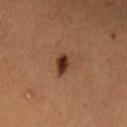notes — catalogued during a skin exam; not biopsied
anatomic site — the mid back
subject — male, aged around 50
tile lighting — cross-polarized
size — ~3 mm (longest diameter)
imaging modality — ~15 mm crop, total-body skin-cancer survey
automated lesion analysis — an area of roughly 5 mm², an eccentricity of roughly 0.7, and a shape-asymmetry score of about 0.3 (0 = symmetric); an average lesion color of about L≈28 a*≈18 b*≈24 (CIELAB) and about 10 CIELAB-L* units darker than the surrounding skin; a border-irregularity rating of about 3/10, a within-lesion color-variation index near 4.5/10, and radial color variation of about 1.5